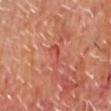Impression:
Captured during whole-body skin photography for melanoma surveillance; the lesion was not biopsied.
Image and clinical context:
On the head or neck. The lesion's longest dimension is about 3 mm. Imaged with cross-polarized lighting. A male subject, approximately 60 years of age. A 15 mm crop from a total-body photograph taken for skin-cancer surveillance. The total-body-photography lesion software estimated an area of roughly 3.5 mm² and two-axis asymmetry of about 0.4. The analysis additionally found about 6 CIELAB-L* units darker than the surrounding skin. And it measured border irregularity of about 5 on a 0–10 scale, a color-variation rating of about 3/10, and peripheral color asymmetry of about 1. The analysis additionally found a classifier nevus-likeness of about 0/100 and a lesion-detection confidence of about 80/100.